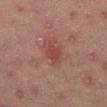A 15 mm close-up extracted from a 3D total-body photography capture. Captured under cross-polarized illumination. About 3 mm across. The subject is a male in their mid-40s. The lesion is on the abdomen.